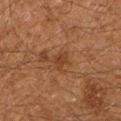workup: no biopsy performed (imaged during a skin exam) | image: ~15 mm tile from a whole-body skin photo | lighting: cross-polarized | location: the left lower leg | lesion size: ~2.5 mm (longest diameter) | subject: male, in their 60s.